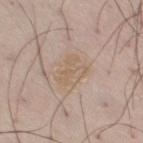| field | value |
|---|---|
| image | ~15 mm tile from a whole-body skin photo |
| site | the right thigh |
| lighting | white-light illumination |
| subject | male, aged 53 to 57 |
| image-analysis metrics | an average lesion color of about L≈60 a*≈14 b*≈28 (CIELAB), roughly 6 lightness units darker than nearby skin, and a lesion-to-skin contrast of about 5 (normalized; higher = more distinct) |
| lesion diameter | ~3.5 mm (longest diameter) |